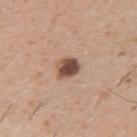Measured at roughly 2.5 mm in maximum diameter.
A 15 mm close-up tile from a total-body photography series done for melanoma screening.
A male patient, aged approximately 50.
The tile uses white-light illumination.
The lesion is located on the right upper arm.